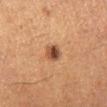notes: total-body-photography surveillance lesion; no biopsy.